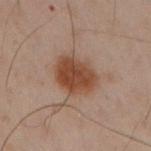| feature | finding |
|---|---|
| follow-up | no biopsy performed (imaged during a skin exam) |
| image | ~15 mm tile from a whole-body skin photo |
| size | ≈4.5 mm |
| body site | the arm |
| tile lighting | cross-polarized |
| patient | male, about 50 years old |